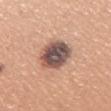Recorded during total-body skin imaging; not selected for excision or biopsy. A 15 mm close-up tile from a total-body photography series done for melanoma screening. The recorded lesion diameter is about 4.5 mm. A female subject aged 28–32. On the right upper arm.